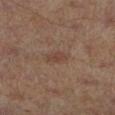lesion size: about 2.5 mm | site: the left lower leg | image: ~15 mm crop, total-body skin-cancer survey | subject: male, aged approximately 65 | lighting: cross-polarized illumination | TBP lesion metrics: a footprint of about 3 mm², a shape eccentricity near 0.9, and a symmetry-axis asymmetry near 0.25; an average lesion color of about L≈36 a*≈17 b*≈23 (CIELAB) and about 6 CIELAB-L* units darker than the surrounding skin.